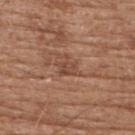No biopsy was performed on this lesion — it was imaged during a full skin examination and was not determined to be concerning.